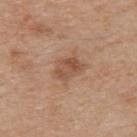Impression: This lesion was catalogued during total-body skin photography and was not selected for biopsy. Acquisition and patient details: The recorded lesion diameter is about 3.5 mm. Imaged with white-light lighting. The lesion is on the upper back. This image is a 15 mm lesion crop taken from a total-body photograph. The patient is a male approximately 70 years of age.